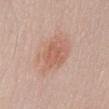<tbp_lesion>
  <biopsy_status>not biopsied; imaged during a skin examination</biopsy_status>
  <site>abdomen</site>
  <patient>
    <sex>male</sex>
    <age_approx>55</age_approx>
  </patient>
  <image>
    <source>total-body photography crop</source>
    <field_of_view_mm>15</field_of_view_mm>
  </image>
</tbp_lesion>